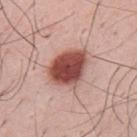On the mid back. Longest diameter approximately 5.5 mm. The total-body-photography lesion software estimated an average lesion color of about L≈49 a*≈26 b*≈26 (CIELAB), about 19 CIELAB-L* units darker than the surrounding skin, and a normalized border contrast of about 12.5. And it measured an automated nevus-likeness rating near 100 out of 100 and lesion-presence confidence of about 100/100. Captured under white-light illumination. A male patient aged approximately 35. Cropped from a total-body skin-imaging series; the visible field is about 15 mm.